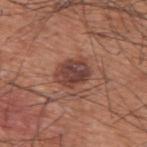This lesion was catalogued during total-body skin photography and was not selected for biopsy. Longest diameter approximately 4 mm. The lesion is located on the upper back. A male subject, aged 58–62. A roughly 15 mm field-of-view crop from a total-body skin photograph. Captured under white-light illumination.